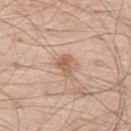The lesion was tiled from a total-body skin photograph and was not biopsied.
A male subject, aged approximately 60.
Cropped from a whole-body photographic skin survey; the tile spans about 15 mm.
The lesion is located on the left thigh.
The lesion's longest dimension is about 3 mm.
This is a white-light tile.
The total-body-photography lesion software estimated an area of roughly 4.5 mm² and a shape eccentricity near 0.65. The analysis additionally found a border-irregularity index near 3.5/10, a color-variation rating of about 2.5/10, and a peripheral color-asymmetry measure near 1. The analysis additionally found a classifier nevus-likeness of about 40/100 and a lesion-detection confidence of about 100/100.